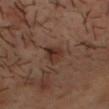workup: catalogued during a skin exam; not biopsied | location: the head or neck | illumination: cross-polarized | patient: male, aged 48 to 52 | diameter: ≈3 mm | image: 15 mm crop, total-body photography.